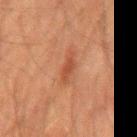body site: the mid back; imaging modality: total-body-photography crop, ~15 mm field of view; subject: male, in their 60s.